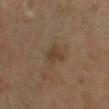Recorded during total-body skin imaging; not selected for excision or biopsy. The lesion-visualizer software estimated an area of roughly 5 mm², an outline eccentricity of about 0.5 (0 = round, 1 = elongated), and a shape-asymmetry score of about 0.35 (0 = symmetric). The analysis additionally found a mean CIELAB color near L≈38 a*≈14 b*≈27 and a normalized border contrast of about 6.5. And it measured a border-irregularity index near 3.5/10 and a peripheral color-asymmetry measure near 0.5. It also reported a nevus-likeness score of about 0/100 and lesion-presence confidence of about 100/100. Imaged with cross-polarized lighting. Located on the right leg. A 15 mm close-up extracted from a 3D total-body photography capture. A male patient, approximately 60 years of age.